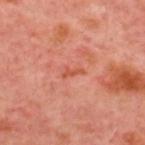{"biopsy_status": "not biopsied; imaged during a skin examination", "patient": {"age_approx": 55}, "image": {"source": "total-body photography crop", "field_of_view_mm": 15}, "site": "upper back"}